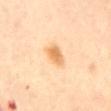Part of a total-body skin-imaging series; this lesion was reviewed on a skin check and was not flagged for biopsy. A 15 mm close-up extracted from a 3D total-body photography capture. The lesion-visualizer software estimated a mean CIELAB color near L≈72 a*≈21 b*≈43, a lesion–skin lightness drop of about 11, and a normalized lesion–skin contrast near 7.5. A female subject, aged 63 to 67. Measured at roughly 3 mm in maximum diameter. This is a cross-polarized tile. The lesion is located on the abdomen.